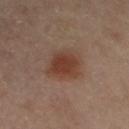biopsy_status: not biopsied; imaged during a skin examination
site: arm
patient:
  sex: female
  age_approx: 65
lighting: cross-polarized
lesion_size:
  long_diameter_mm_approx: 5.0
image:
  source: total-body photography crop
  field_of_view_mm: 15